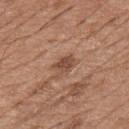<case>
<biopsy_status>not biopsied; imaged during a skin examination</biopsy_status>
</case>